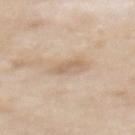{"biopsy_status": "not biopsied; imaged during a skin examination", "image": {"source": "total-body photography crop", "field_of_view_mm": 15}, "site": "mid back", "patient": {"sex": "female", "age_approx": 60}}